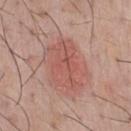Part of a total-body skin-imaging series; this lesion was reviewed on a skin check and was not flagged for biopsy.
About 6.5 mm across.
A region of skin cropped from a whole-body photographic capture, roughly 15 mm wide.
Located on the chest.
A male patient, about 45 years old.
This is a white-light tile.